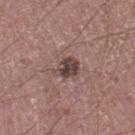biopsy status: total-body-photography surveillance lesion; no biopsy
lighting: white-light
automated lesion analysis: an area of roughly 5.5 mm², an outline eccentricity of about 0.55 (0 = round, 1 = elongated), and a symmetry-axis asymmetry near 0.25
subject: male, approximately 60 years of age
lesion size: about 3 mm
acquisition: ~15 mm crop, total-body skin-cancer survey
body site: the leg